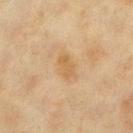Captured during whole-body skin photography for melanoma surveillance; the lesion was not biopsied.
A female subject, aged around 40.
Cropped from a whole-body photographic skin survey; the tile spans about 15 mm.
The lesion is located on the left lower leg.